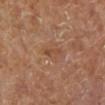Imaged during a routine full-body skin examination; the lesion was not biopsied and no histopathology is available. From the left lower leg. Cropped from a whole-body photographic skin survey; the tile spans about 15 mm. Measured at roughly 2.5 mm in maximum diameter. The patient is female.